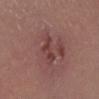Q: Was this lesion biopsied?
A: no biopsy performed (imaged during a skin exam)
Q: How was this image acquired?
A: ~15 mm tile from a whole-body skin photo
Q: Lesion location?
A: the left lower leg
Q: What is the lesion's diameter?
A: ≈3.5 mm
Q: What are the patient's age and sex?
A: female, aged 18 to 22